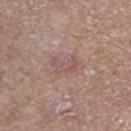Notes:
* notes: catalogued during a skin exam; not biopsied
* subject: male, about 85 years old or older
* size: about 3 mm
* body site: the leg
* acquisition: total-body-photography crop, ~15 mm field of view
* lighting: white-light